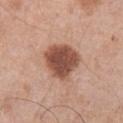Clinical impression:
Part of a total-body skin-imaging series; this lesion was reviewed on a skin check and was not flagged for biopsy.
Image and clinical context:
Imaged with white-light lighting. A male patient aged approximately 70. Cropped from a whole-body photographic skin survey; the tile spans about 15 mm. The total-body-photography lesion software estimated a lesion color around L≈50 a*≈23 b*≈28 in CIELAB, about 16 CIELAB-L* units darker than the surrounding skin, and a normalized lesion–skin contrast near 11. The analysis additionally found border irregularity of about 2 on a 0–10 scale and a color-variation rating of about 4/10. It also reported an automated nevus-likeness rating near 80 out of 100. On the abdomen.